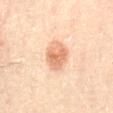  biopsy_status: not biopsied; imaged during a skin examination
  lighting: cross-polarized
  image:
    source: total-body photography crop
    field_of_view_mm: 15
  patient:
    sex: male
    age_approx: 85+
  automated_metrics:
    area_mm2_approx: 8.5
    eccentricity: 0.55
    shape_asymmetry: 0.15
    nevus_likeness_0_100: 95
    lesion_detection_confidence_0_100: 100
  site: abdomen